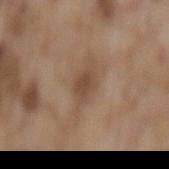lighting: white-light
lesion_size:
  long_diameter_mm_approx: 2.5
site: mid back
image:
  source: total-body photography crop
  field_of_view_mm: 15
patient:
  sex: male
  age_approx: 75